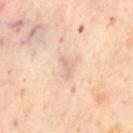<case>
  <biopsy_status>not biopsied; imaged during a skin examination</biopsy_status>
</case>